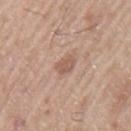Automated image analysis of the tile measured a footprint of about 4 mm², an eccentricity of roughly 0.7, and a shape-asymmetry score of about 0.25 (0 = symmetric). And it measured a detector confidence of about 100 out of 100 that the crop contains a lesion.
A male patient, aged approximately 70.
Imaged with white-light lighting.
A 15 mm close-up tile from a total-body photography series done for melanoma screening.
The lesion is located on the left upper arm.
About 2.5 mm across.